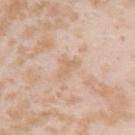- notes — catalogued during a skin exam; not biopsied
- lighting — white-light
- image source — ~15 mm tile from a whole-body skin photo
- TBP lesion metrics — a lesion area of about 4.5 mm² and an outline eccentricity of about 0.7 (0 = round, 1 = elongated); a lesion color around L≈67 a*≈17 b*≈32 in CIELAB, roughly 6 lightness units darker than nearby skin, and a lesion-to-skin contrast of about 5 (normalized; higher = more distinct); an automated nevus-likeness rating near 0 out of 100 and a detector confidence of about 100 out of 100 that the crop contains a lesion
- patient — female, in their mid-20s
- location — the right upper arm